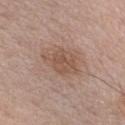Impression: Part of a total-body skin-imaging series; this lesion was reviewed on a skin check and was not flagged for biopsy. Image and clinical context: A roughly 15 mm field-of-view crop from a total-body skin photograph. The subject is a male approximately 55 years of age. The lesion is located on the chest.